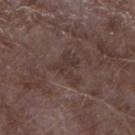Findings:
– location — the left forearm
– subject — male, about 65 years old
– lesion size — ~4 mm (longest diameter)
– imaging modality — total-body-photography crop, ~15 mm field of view
– image-analysis metrics — a footprint of about 7 mm², a shape eccentricity near 0.6, and a shape-asymmetry score of about 0.6 (0 = symmetric); a border-irregularity index near 7.5/10, a color-variation rating of about 2.5/10, and a peripheral color-asymmetry measure near 1; a classifier nevus-likeness of about 0/100 and a detector confidence of about 80 out of 100 that the crop contains a lesion
– lighting — white-light illumination